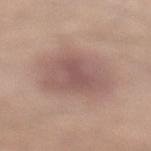Recorded during total-body skin imaging; not selected for excision or biopsy.
On the left lower leg.
The patient is a male approximately 30 years of age.
A close-up tile cropped from a whole-body skin photograph, about 15 mm across.
The tile uses white-light illumination.
Longest diameter approximately 7.5 mm.